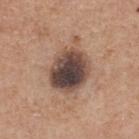biopsy status = no biopsy performed (imaged during a skin exam) | lesion size = about 6.5 mm | image-analysis metrics = about 17 CIELAB-L* units darker than the surrounding skin and a normalized border contrast of about 13; a border-irregularity index near 3/10, a color-variation rating of about 9.5/10, and a peripheral color-asymmetry measure near 3; an automated nevus-likeness rating near 5 out of 100 and lesion-presence confidence of about 100/100 | body site = the back | illumination = white-light | image = 15 mm crop, total-body photography | patient = male, in their mid- to late 60s.